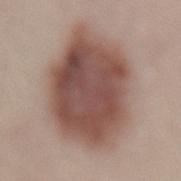This lesion was catalogued during total-body skin photography and was not selected for biopsy. This image is a 15 mm lesion crop taken from a total-body photograph. The patient is a female roughly 50 years of age. Located on the lower back.